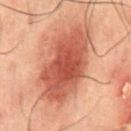Assessment:
Recorded during total-body skin imaging; not selected for excision or biopsy.
Image and clinical context:
This is a cross-polarized tile. The recorded lesion diameter is about 9 mm. A roughly 15 mm field-of-view crop from a total-body skin photograph. The patient is a male in their 50s. Located on the abdomen.